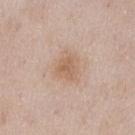Impression: No biopsy was performed on this lesion — it was imaged during a full skin examination and was not determined to be concerning. Context: Imaged with white-light lighting. A 15 mm close-up tile from a total-body photography series done for melanoma screening. On the front of the torso. About 3 mm across. The patient is a male approximately 55 years of age.